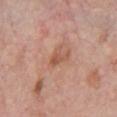Q: Is there a histopathology result?
A: total-body-photography surveillance lesion; no biopsy
Q: What is the imaging modality?
A: ~15 mm tile from a whole-body skin photo
Q: What is the anatomic site?
A: the chest
Q: Who is the patient?
A: male, roughly 60 years of age
Q: Automated lesion metrics?
A: an average lesion color of about L≈55 a*≈23 b*≈31 (CIELAB) and roughly 8 lightness units darker than nearby skin; a border-irregularity rating of about 4/10, a color-variation rating of about 1.5/10, and a peripheral color-asymmetry measure near 0.5
Q: Illumination type?
A: white-light